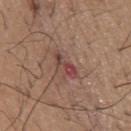The lesion was photographed on a routine skin check and not biopsied; there is no pathology result. Cropped from a total-body skin-imaging series; the visible field is about 15 mm. On the chest. A male subject approximately 65 years of age.